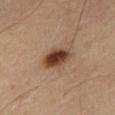Clinical impression:
The lesion was photographed on a routine skin check and not biopsied; there is no pathology result.
Image and clinical context:
A roughly 15 mm field-of-view crop from a total-body skin photograph. A male subject, aged around 65.